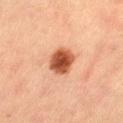This lesion was catalogued during total-body skin photography and was not selected for biopsy.
Captured under cross-polarized illumination.
A female patient in their 50s.
The recorded lesion diameter is about 4 mm.
A 15 mm crop from a total-body photograph taken for skin-cancer surveillance.